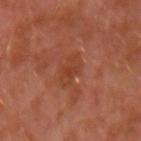Findings:
- biopsy status — imaged on a skin check; not biopsied
- imaging modality — ~15 mm tile from a whole-body skin photo
- patient — male, roughly 30 years of age
- site — the left upper arm